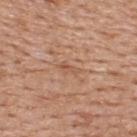Q: Is there a histopathology result?
A: total-body-photography surveillance lesion; no biopsy
Q: What are the patient's age and sex?
A: male, about 60 years old
Q: Lesion location?
A: the upper back
Q: What is the lesion's diameter?
A: ≈2.5 mm
Q: What is the imaging modality?
A: ~15 mm crop, total-body skin-cancer survey